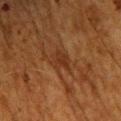biopsy status = catalogued during a skin exam; not biopsied | illumination = cross-polarized | size = ≈3.5 mm | image-analysis metrics = a lesion area of about 5.5 mm² and a symmetry-axis asymmetry near 0.25; a border-irregularity rating of about 3/10, a within-lesion color-variation index near 2/10, and a peripheral color-asymmetry measure near 0.5 | image = ~15 mm crop, total-body skin-cancer survey | anatomic site = the upper back | subject = male, about 60 years old.